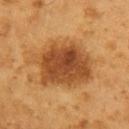Findings:
- workup — no biopsy performed (imaged during a skin exam)
- patient — male, about 60 years old
- anatomic site — the right upper arm
- image source — ~15 mm crop, total-body skin-cancer survey
- image-analysis metrics — an area of roughly 31 mm², a shape eccentricity near 0.8, and two-axis asymmetry of about 0.25; border irregularity of about 3 on a 0–10 scale, internal color variation of about 6.5 on a 0–10 scale, and radial color variation of about 2; a classifier nevus-likeness of about 95/100 and a lesion-detection confidence of about 100/100
- size — ~8.5 mm (longest diameter)